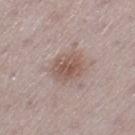Assessment:
Imaged during a routine full-body skin examination; the lesion was not biopsied and no histopathology is available.
Image and clinical context:
Imaged with white-light lighting. On the left thigh. A 15 mm crop from a total-body photograph taken for skin-cancer surveillance. The patient is a female roughly 30 years of age. Longest diameter approximately 4 mm.